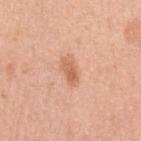Imaged during a routine full-body skin examination; the lesion was not biopsied and no histopathology is available.